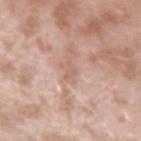Impression: Captured during whole-body skin photography for melanoma surveillance; the lesion was not biopsied. Background: From the left forearm. Automated image analysis of the tile measured a nevus-likeness score of about 0/100 and lesion-presence confidence of about 95/100. The patient is a male aged 38 to 42. This image is a 15 mm lesion crop taken from a total-body photograph. Captured under white-light illumination. Measured at roughly 2.5 mm in maximum diameter.